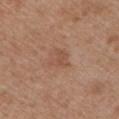Clinical impression:
The lesion was photographed on a routine skin check and not biopsied; there is no pathology result.
Image and clinical context:
From the chest. Cropped from a total-body skin-imaging series; the visible field is about 15 mm. The patient is a male approximately 65 years of age.